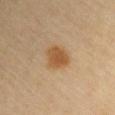Impression:
Captured during whole-body skin photography for melanoma surveillance; the lesion was not biopsied.
Acquisition and patient details:
The subject is a male aged approximately 60. On the left upper arm. Captured under cross-polarized illumination. The lesion's longest dimension is about 3 mm. Cropped from a whole-body photographic skin survey; the tile spans about 15 mm.